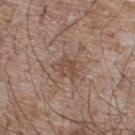The lesion was photographed on a routine skin check and not biopsied; there is no pathology result.
Located on the upper back.
Approximately 3 mm at its widest.
A lesion tile, about 15 mm wide, cut from a 3D total-body photograph.
A male subject, approximately 65 years of age.
Imaged with white-light lighting.